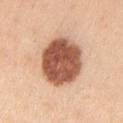Part of a total-body skin-imaging series; this lesion was reviewed on a skin check and was not flagged for biopsy. A close-up tile cropped from a whole-body skin photograph, about 15 mm across. A male patient, about 35 years old. Imaged with white-light lighting. Approximately 6.5 mm at its widest. The lesion is on the left upper arm.